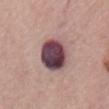No biopsy was performed on this lesion — it was imaged during a full skin examination and was not determined to be concerning. Longest diameter approximately 4.5 mm. From the chest. Automated tile analysis of the lesion measured an average lesion color of about L≈44 a*≈21 b*≈13 (CIELAB), roughly 21 lightness units darker than nearby skin, and a lesion-to-skin contrast of about 16 (normalized; higher = more distinct). A roughly 15 mm field-of-view crop from a total-body skin photograph. A female patient, about 70 years old.